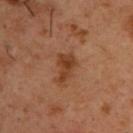biopsy status: imaged on a skin check; not biopsied
image source: ~15 mm crop, total-body skin-cancer survey
subject: male, approximately 55 years of age
location: the upper back